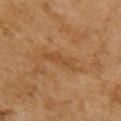Background: This is a cross-polarized tile. Automated tile analysis of the lesion measured an eccentricity of roughly 0.95 and a symmetry-axis asymmetry near 0.4. And it measured a border-irregularity index near 5/10 and a peripheral color-asymmetry measure near 0. A 15 mm close-up tile from a total-body photography series done for melanoma screening. The lesion is on the right upper arm. Approximately 4.5 mm at its widest. A female subject aged approximately 60.